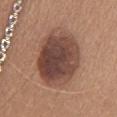The lesion was tiled from a total-body skin photograph and was not biopsied.
A female patient, aged 33 to 37.
A lesion tile, about 15 mm wide, cut from a 3D total-body photograph.
Located on the upper back.